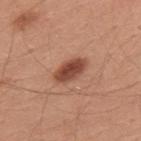Impression: The lesion was tiled from a total-body skin photograph and was not biopsied. Acquisition and patient details: The lesion's longest dimension is about 4 mm. From the upper back. Imaged with white-light lighting. Cropped from a whole-body photographic skin survey; the tile spans about 15 mm. The subject is a male roughly 30 years of age.